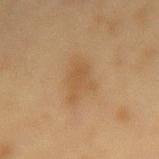biopsy status=catalogued during a skin exam; not biopsied | TBP lesion metrics=an automated nevus-likeness rating near 0 out of 100 and lesion-presence confidence of about 100/100 | image source=~15 mm crop, total-body skin-cancer survey | tile lighting=cross-polarized illumination | patient=female, about 55 years old | body site=the mid back.